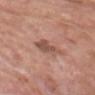Clinical impression: No biopsy was performed on this lesion — it was imaged during a full skin examination and was not determined to be concerning. Context: A male subject, aged 58–62. The lesion is located on the head or neck. A roughly 15 mm field-of-view crop from a total-body skin photograph.